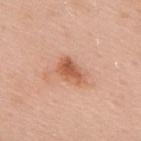| feature | finding |
|---|---|
| follow-up | no biopsy performed (imaged during a skin exam) |
| acquisition | 15 mm crop, total-body photography |
| location | the upper back |
| subject | male, approximately 55 years of age |